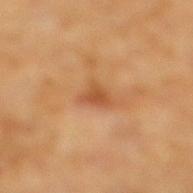biopsy_status: not biopsied; imaged during a skin examination
patient:
  sex: male
  age_approx: 60
lesion_size:
  long_diameter_mm_approx: 2.5
image:
  source: total-body photography crop
  field_of_view_mm: 15
automated_metrics:
  border_irregularity_0_10: 3.5
  color_variation_0_10: 2.0
  peripheral_color_asymmetry: 0.5
  nevus_likeness_0_100: 0
  lesion_detection_confidence_0_100: 100
site: right lower leg
lighting: cross-polarized